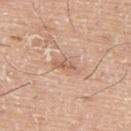Q: Was a biopsy performed?
A: total-body-photography surveillance lesion; no biopsy
Q: Lesion size?
A: ≈3.5 mm
Q: What lighting was used for the tile?
A: white-light illumination
Q: What is the anatomic site?
A: the upper back
Q: What is the imaging modality?
A: ~15 mm crop, total-body skin-cancer survey
Q: Who is the patient?
A: male, aged 73–77